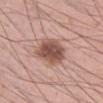{"biopsy_status": "not biopsied; imaged during a skin examination", "lighting": "white-light", "site": "right thigh", "patient": {"sex": "male", "age_approx": 55}, "image": {"source": "total-body photography crop", "field_of_view_mm": 15}}